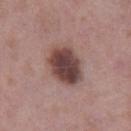{"biopsy_status": "not biopsied; imaged during a skin examination", "site": "right lower leg", "lesion_size": {"long_diameter_mm_approx": 5.0}, "automated_metrics": {"area_mm2_approx": 15.0, "eccentricity": 0.65, "shape_asymmetry": 0.15, "nevus_likeness_0_100": 40, "lesion_detection_confidence_0_100": 100}, "lighting": "white-light", "patient": {"sex": "male", "age_approx": 55}, "image": {"source": "total-body photography crop", "field_of_view_mm": 15}}